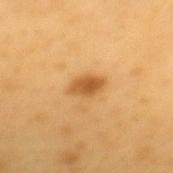Assessment: This lesion was catalogued during total-body skin photography and was not selected for biopsy. Acquisition and patient details: The lesion's longest dimension is about 3.5 mm. A male subject roughly 60 years of age. Automated tile analysis of the lesion measured a lesion area of about 5.5 mm², an eccentricity of roughly 0.8, and a symmetry-axis asymmetry near 0.25. The software also gave an average lesion color of about L≈57 a*≈23 b*≈45 (CIELAB) and about 12 CIELAB-L* units darker than the surrounding skin. The software also gave a border-irregularity index near 2.5/10, internal color variation of about 4 on a 0–10 scale, and radial color variation of about 1. It also reported a classifier nevus-likeness of about 95/100 and a lesion-detection confidence of about 100/100. This image is a 15 mm lesion crop taken from a total-body photograph. On the mid back. Imaged with cross-polarized lighting.